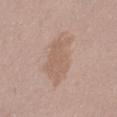| field | value |
|---|---|
| notes | catalogued during a skin exam; not biopsied |
| image-analysis metrics | an average lesion color of about L≈60 a*≈16 b*≈27 (CIELAB), roughly 7 lightness units darker than nearby skin, and a normalized lesion–skin contrast near 5; internal color variation of about 2 on a 0–10 scale and a peripheral color-asymmetry measure near 0.5; a classifier nevus-likeness of about 0/100 and lesion-presence confidence of about 100/100 |
| subject | female, aged approximately 20 |
| illumination | white-light |
| lesion size | about 6.5 mm |
| image source | total-body-photography crop, ~15 mm field of view |
| site | the leg |